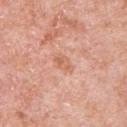This lesion was catalogued during total-body skin photography and was not selected for biopsy. The tile uses white-light illumination. On the left upper arm. A close-up tile cropped from a whole-body skin photograph, about 15 mm across. An algorithmic analysis of the crop reported a footprint of about 3.5 mm² and an outline eccentricity of about 0.8 (0 = round, 1 = elongated). The analysis additionally found a lesion color around L≈63 a*≈25 b*≈33 in CIELAB, about 7 CIELAB-L* units darker than the surrounding skin, and a normalized lesion–skin contrast near 5.5. The patient is a male aged approximately 80. The lesion's longest dimension is about 2.5 mm.